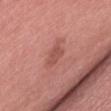This lesion was catalogued during total-body skin photography and was not selected for biopsy. Imaged with white-light lighting. This image is a 15 mm lesion crop taken from a total-body photograph. Approximately 2.5 mm at its widest. On the left thigh. A female patient in their 70s.